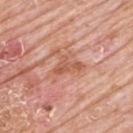notes — catalogued during a skin exam; not biopsied | automated metrics — an area of roughly 7 mm², an outline eccentricity of about 0.8 (0 = round, 1 = elongated), and two-axis asymmetry of about 0.55; a border-irregularity index near 7/10 and internal color variation of about 3 on a 0–10 scale; a nevus-likeness score of about 0/100 and a lesion-detection confidence of about 100/100 | body site — the upper back | lighting — white-light illumination | patient — male, in their 80s | diameter — ~4 mm (longest diameter) | imaging modality — total-body-photography crop, ~15 mm field of view.